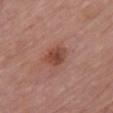Case summary:
– follow-up: total-body-photography surveillance lesion; no biopsy
– image-analysis metrics: a mean CIELAB color near L≈46 a*≈24 b*≈28, about 10 CIELAB-L* units darker than the surrounding skin, and a lesion-to-skin contrast of about 7.5 (normalized; higher = more distinct); a classifier nevus-likeness of about 75/100 and lesion-presence confidence of about 100/100
– size: ~3 mm (longest diameter)
– tile lighting: white-light
– body site: the chest
– image source: ~15 mm tile from a whole-body skin photo
– subject: female, aged around 65